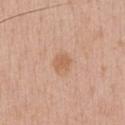The lesion was tiled from a total-body skin photograph and was not biopsied. A male patient, in their 30s. The lesion is located on the chest. A roughly 15 mm field-of-view crop from a total-body skin photograph.